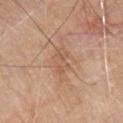Imaged during a routine full-body skin examination; the lesion was not biopsied and no histopathology is available. This image is a 15 mm lesion crop taken from a total-body photograph. A male subject, approximately 80 years of age. Measured at roughly 3 mm in maximum diameter. The lesion is located on the chest.